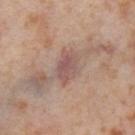No biopsy was performed on this lesion — it was imaged during a full skin examination and was not determined to be concerning. The subject is a female roughly 55 years of age. From the right thigh. A 15 mm close-up tile from a total-body photography series done for melanoma screening.